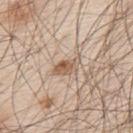{
  "biopsy_status": "not biopsied; imaged during a skin examination",
  "lesion_size": {
    "long_diameter_mm_approx": 3.5
  },
  "patient": {
    "sex": "male",
    "age_approx": 80
  },
  "image": {
    "source": "total-body photography crop",
    "field_of_view_mm": 15
  },
  "lighting": "white-light",
  "site": "upper back"
}